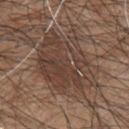Case summary:
- anatomic site · the chest
- subject · male, in their mid-40s
- acquisition · 15 mm crop, total-body photography
- lesion size · ≈8 mm